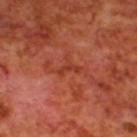Findings:
• follow-up — imaged on a skin check; not biopsied
• anatomic site — the back
• image — ~15 mm tile from a whole-body skin photo
• patient — male, approximately 70 years of age